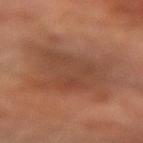Q: Is there a histopathology result?
A: imaged on a skin check; not biopsied
Q: Automated lesion metrics?
A: an area of roughly 24 mm² and a shape-asymmetry score of about 0.4 (0 = symmetric); a mean CIELAB color near L≈43 a*≈22 b*≈29, roughly 7 lightness units darker than nearby skin, and a lesion-to-skin contrast of about 5.5 (normalized; higher = more distinct); internal color variation of about 3 on a 0–10 scale and peripheral color asymmetry of about 1; a classifier nevus-likeness of about 0/100 and lesion-presence confidence of about 100/100
Q: Lesion size?
A: about 6 mm
Q: What lighting was used for the tile?
A: cross-polarized
Q: Lesion location?
A: the arm
Q: What kind of image is this?
A: 15 mm crop, total-body photography
Q: What are the patient's age and sex?
A: male, in their 70s